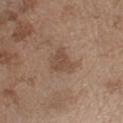Findings:
– workup: catalogued during a skin exam; not biopsied
– TBP lesion metrics: an area of roughly 5.5 mm² and an outline eccentricity of about 0.35 (0 = round, 1 = elongated); a border-irregularity rating of about 4/10, a color-variation rating of about 2/10, and peripheral color asymmetry of about 1
– illumination: white-light illumination
– anatomic site: the arm
– diameter: about 3 mm
– image source: ~15 mm tile from a whole-body skin photo
– subject: male, aged approximately 70